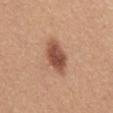Imaged during a routine full-body skin examination; the lesion was not biopsied and no histopathology is available. The lesion is located on the mid back. Measured at roughly 4.5 mm in maximum diameter. A close-up tile cropped from a whole-body skin photograph, about 15 mm across. The subject is a female aged around 55.